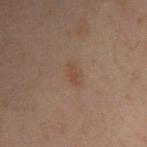Part of a total-body skin-imaging series; this lesion was reviewed on a skin check and was not flagged for biopsy.